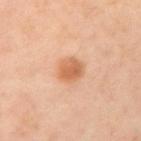follow-up — catalogued during a skin exam; not biopsied